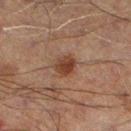Clinical impression:
The lesion was tiled from a total-body skin photograph and was not biopsied.
Background:
On the left lower leg. A male subject, approximately 60 years of age. The tile uses cross-polarized illumination. A 15 mm close-up extracted from a 3D total-body photography capture.